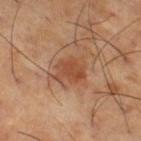The lesion was photographed on a routine skin check and not biopsied; there is no pathology result. This image is a 15 mm lesion crop taken from a total-body photograph. A male subject, roughly 65 years of age. The tile uses cross-polarized illumination. From the right thigh. Measured at roughly 3.5 mm in maximum diameter.